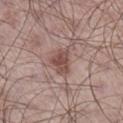This lesion was catalogued during total-body skin photography and was not selected for biopsy. On the right lower leg. A 15 mm close-up extracted from a 3D total-body photography capture. The subject is a male about 55 years old. Longest diameter approximately 3 mm. This is a white-light tile.